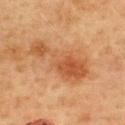Clinical impression: Captured during whole-body skin photography for melanoma surveillance; the lesion was not biopsied. Image and clinical context: The lesion's longest dimension is about 7.5 mm. This is a cross-polarized tile. The patient is a female about 60 years old. From the upper back. This image is a 15 mm lesion crop taken from a total-body photograph.